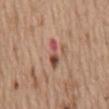This image is a 15 mm lesion crop taken from a total-body photograph. Measured at roughly 3.5 mm in maximum diameter. The total-body-photography lesion software estimated a footprint of about 4 mm², an eccentricity of roughly 0.95, and a symmetry-axis asymmetry near 0.45. Located on the mid back. A male subject in their 70s. Captured under white-light illumination.